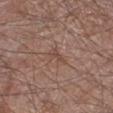This lesion was catalogued during total-body skin photography and was not selected for biopsy.
The lesion-visualizer software estimated a lesion color around L≈47 a*≈18 b*≈25 in CIELAB and a normalized border contrast of about 5. The analysis additionally found a border-irregularity rating of about 5/10, internal color variation of about 0.5 on a 0–10 scale, and radial color variation of about 0. The analysis additionally found a detector confidence of about 90 out of 100 that the crop contains a lesion.
A male patient, aged 58–62.
The lesion is located on the left lower leg.
Imaged with white-light lighting.
Cropped from a whole-body photographic skin survey; the tile spans about 15 mm.
Measured at roughly 3 mm in maximum diameter.